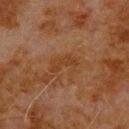The lesion was photographed on a routine skin check and not biopsied; there is no pathology result. The tile uses cross-polarized illumination. On the upper back. Approximately 3.5 mm at its widest. An algorithmic analysis of the crop reported a footprint of about 5.5 mm² and a symmetry-axis asymmetry near 0.45. And it measured roughly 5 lightness units darker than nearby skin and a lesion-to-skin contrast of about 6 (normalized; higher = more distinct). The analysis additionally found a classifier nevus-likeness of about 0/100. This image is a 15 mm lesion crop taken from a total-body photograph. The patient is a male aged approximately 80.